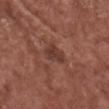The lesion was photographed on a routine skin check and not biopsied; there is no pathology result. A male subject approximately 75 years of age. A close-up tile cropped from a whole-body skin photograph, about 15 mm across. The tile uses white-light illumination. The lesion is on the chest. Approximately 3 mm at its widest.